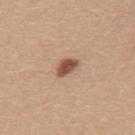Part of a total-body skin-imaging series; this lesion was reviewed on a skin check and was not flagged for biopsy.
The recorded lesion diameter is about 3 mm.
A close-up tile cropped from a whole-body skin photograph, about 15 mm across.
The subject is a female approximately 25 years of age.
The lesion is located on the back.